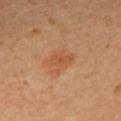A 15 mm close-up extracted from a 3D total-body photography capture. A female patient about 30 years old. The lesion is located on the back.